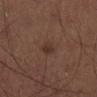Q: Is there a histopathology result?
A: no biopsy performed (imaged during a skin exam)
Q: How was the tile lit?
A: cross-polarized
Q: How was this image acquired?
A: ~15 mm tile from a whole-body skin photo
Q: What are the patient's age and sex?
A: male, in their mid-60s
Q: What is the lesion's diameter?
A: about 2.5 mm
Q: Automated lesion metrics?
A: roughly 5 lightness units darker than nearby skin and a normalized border contrast of about 6; lesion-presence confidence of about 100/100
Q: Where on the body is the lesion?
A: the left thigh